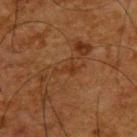biopsy status = imaged on a skin check; not biopsied
image = ~15 mm crop, total-body skin-cancer survey
diameter = about 3 mm
automated lesion analysis = a border-irregularity index near 8/10, a color-variation rating of about 0/10, and radial color variation of about 0
illumination = cross-polarized
anatomic site = the upper back
patient = male, aged 63–67